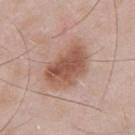Clinical impression: Recorded during total-body skin imaging; not selected for excision or biopsy. Image and clinical context: A male subject aged 53 to 57. Measured at roughly 5.5 mm in maximum diameter. Located on the front of the torso. The total-body-photography lesion software estimated an average lesion color of about L≈54 a*≈21 b*≈28 (CIELAB) and a normalized lesion–skin contrast near 8.5. The analysis additionally found a border-irregularity rating of about 3/10 and a peripheral color-asymmetry measure near 1.5. It also reported a classifier nevus-likeness of about 90/100 and a detector confidence of about 100 out of 100 that the crop contains a lesion. A close-up tile cropped from a whole-body skin photograph, about 15 mm across.